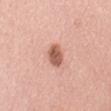Recorded during total-body skin imaging; not selected for excision or biopsy. A female subject, aged around 65. The tile uses white-light illumination. The lesion is located on the back. Automated image analysis of the tile measured a lesion area of about 5.5 mm² and an outline eccentricity of about 0.75 (0 = round, 1 = elongated). The software also gave border irregularity of about 1.5 on a 0–10 scale and a peripheral color-asymmetry measure near 1. Approximately 3 mm at its widest. Cropped from a whole-body photographic skin survey; the tile spans about 15 mm.